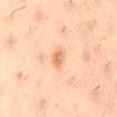Findings:
– follow-up — total-body-photography surveillance lesion; no biopsy
– imaging modality — ~15 mm crop, total-body skin-cancer survey
– tile lighting — cross-polarized illumination
– anatomic site — the back
– automated metrics — a lesion color around L≈69 a*≈23 b*≈40 in CIELAB, roughly 11 lightness units darker than nearby skin, and a lesion-to-skin contrast of about 7.5 (normalized; higher = more distinct)
– diameter — ~2.5 mm (longest diameter)
– patient — male, aged approximately 55